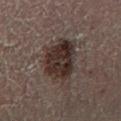Q: Is there a histopathology result?
A: total-body-photography surveillance lesion; no biopsy